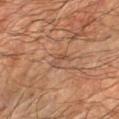Q: Was this lesion biopsied?
A: imaged on a skin check; not biopsied
Q: What is the anatomic site?
A: the left forearm
Q: Automated lesion metrics?
A: a lesion area of about 2.5 mm² and a shape eccentricity near 0.9
Q: What is the imaging modality?
A: total-body-photography crop, ~15 mm field of view
Q: What are the patient's age and sex?
A: male, approximately 60 years of age
Q: Lesion size?
A: ≈2.5 mm
Q: Illumination type?
A: cross-polarized